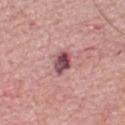Part of a total-body skin-imaging series; this lesion was reviewed on a skin check and was not flagged for biopsy.
A male patient aged 63–67.
Captured under white-light illumination.
The recorded lesion diameter is about 3.5 mm.
Automated tile analysis of the lesion measured an outline eccentricity of about 0.8 (0 = round, 1 = elongated) and a symmetry-axis asymmetry near 0.3. It also reported an average lesion color of about L≈49 a*≈27 b*≈17 (CIELAB), about 17 CIELAB-L* units darker than the surrounding skin, and a normalized border contrast of about 11.5. The software also gave internal color variation of about 8 on a 0–10 scale and peripheral color asymmetry of about 2.5. It also reported a nevus-likeness score of about 60/100 and a lesion-detection confidence of about 100/100.
Cropped from a total-body skin-imaging series; the visible field is about 15 mm.
On the front of the torso.